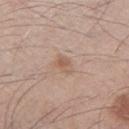Q: Is there a histopathology result?
A: imaged on a skin check; not biopsied
Q: What lighting was used for the tile?
A: white-light
Q: What is the imaging modality?
A: 15 mm crop, total-body photography
Q: Patient demographics?
A: male, in their mid- to late 40s
Q: What is the anatomic site?
A: the left thigh
Q: How large is the lesion?
A: ≈2.5 mm